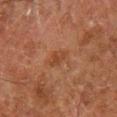Part of a total-body skin-imaging series; this lesion was reviewed on a skin check and was not flagged for biopsy.
A close-up tile cropped from a whole-body skin photograph, about 15 mm across.
A male subject, aged 78–82.
Measured at roughly 3 mm in maximum diameter.
Located on the right lower leg.